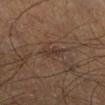Assessment: Part of a total-body skin-imaging series; this lesion was reviewed on a skin check and was not flagged for biopsy. Image and clinical context: A 15 mm close-up extracted from a 3D total-body photography capture. The lesion is located on the left lower leg. The recorded lesion diameter is about 3 mm. Automated image analysis of the tile measured a footprint of about 3 mm², a shape eccentricity near 0.9, and a symmetry-axis asymmetry near 0.2. It also reported an average lesion color of about L≈33 a*≈15 b*≈23 (CIELAB), a lesion–skin lightness drop of about 6, and a normalized lesion–skin contrast near 6. Captured under cross-polarized illumination. The patient is a male about 60 years old.